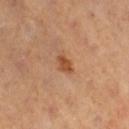{"biopsy_status": "not biopsied; imaged during a skin examination", "automated_metrics": {"area_mm2_approx": 3.5, "eccentricity": 0.75, "shape_asymmetry": 0.25, "cielab_L": 50, "cielab_a": 24, "cielab_b": 36, "vs_skin_darker_L": 10.0, "vs_skin_contrast_norm": 8.0, "lesion_detection_confidence_0_100": 100}, "lesion_size": {"long_diameter_mm_approx": 2.5}, "site": "leg", "lighting": "cross-polarized", "patient": {"sex": "female", "age_approx": 40}, "image": {"source": "total-body photography crop", "field_of_view_mm": 15}}